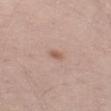notes = catalogued during a skin exam; not biopsied
subject = male, in their mid-40s
imaging modality = 15 mm crop, total-body photography
location = the left thigh